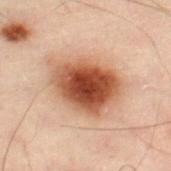The lesion was tiled from a total-body skin photograph and was not biopsied. A male subject, in their 50s. Approximately 7.5 mm at its widest. Located on the left leg. A 15 mm close-up extracted from a 3D total-body photography capture. Imaged with cross-polarized lighting.